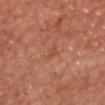Assessment:
Recorded during total-body skin imaging; not selected for excision or biopsy.